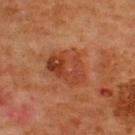Q: Was this lesion biopsied?
A: catalogued during a skin exam; not biopsied
Q: Lesion size?
A: about 5.5 mm
Q: Patient demographics?
A: male, roughly 65 years of age
Q: How was this image acquired?
A: 15 mm crop, total-body photography
Q: Lesion location?
A: the upper back
Q: What lighting was used for the tile?
A: cross-polarized